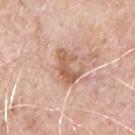Notes:
- workup · total-body-photography surveillance lesion; no biopsy
- tile lighting · white-light illumination
- body site · the front of the torso
- patient · male, aged 68–72
- image source · 15 mm crop, total-body photography